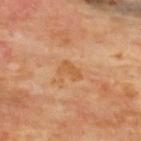Clinical impression: Imaged during a routine full-body skin examination; the lesion was not biopsied and no histopathology is available. Background: The patient is a male in their mid-60s. Measured at roughly 2.5 mm in maximum diameter. On the back. Cropped from a total-body skin-imaging series; the visible field is about 15 mm. This is a cross-polarized tile. Automated tile analysis of the lesion measured a footprint of about 3.5 mm², an outline eccentricity of about 0.85 (0 = round, 1 = elongated), and two-axis asymmetry of about 0.3. It also reported an average lesion color of about L≈54 a*≈23 b*≈39 (CIELAB) and a normalized border contrast of about 6. The software also gave a classifier nevus-likeness of about 0/100 and a detector confidence of about 100 out of 100 that the crop contains a lesion.